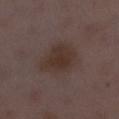follow-up: imaged on a skin check; not biopsied | body site: the leg | subject: female, aged approximately 30 | image-analysis metrics: a footprint of about 15 mm², an eccentricity of roughly 0.7, and a symmetry-axis asymmetry near 0.2; a mean CIELAB color near L≈32 a*≈14 b*≈20, roughly 6 lightness units darker than nearby skin, and a normalized border contrast of about 7.5; a border-irregularity index near 2.5/10 and internal color variation of about 2.5 on a 0–10 scale; an automated nevus-likeness rating near 25 out of 100 and lesion-presence confidence of about 100/100 | lighting: white-light illumination | image: ~15 mm crop, total-body skin-cancer survey | lesion diameter: ~5 mm (longest diameter).